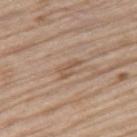Q: Is there a histopathology result?
A: catalogued during a skin exam; not biopsied
Q: Illumination type?
A: white-light
Q: Who is the patient?
A: male, aged 68 to 72
Q: Automated lesion metrics?
A: a mean CIELAB color near L≈55 a*≈17 b*≈30 and a lesion–skin lightness drop of about 7; a peripheral color-asymmetry measure near 0.5; an automated nevus-likeness rating near 0 out of 100 and lesion-presence confidence of about 85/100
Q: How large is the lesion?
A: ≈3 mm
Q: What kind of image is this?
A: ~15 mm tile from a whole-body skin photo
Q: What is the anatomic site?
A: the right thigh